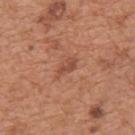* follow-up — catalogued during a skin exam; not biopsied
* lighting — white-light illumination
* body site — the mid back
* lesion size — ≈2.5 mm
* image — ~15 mm tile from a whole-body skin photo
* patient — male, aged 63–67